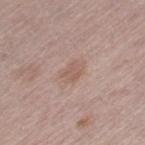Clinical impression: Imaged during a routine full-body skin examination; the lesion was not biopsied and no histopathology is available. Acquisition and patient details: The tile uses white-light illumination. Automated tile analysis of the lesion measured a footprint of about 4.5 mm², a shape eccentricity near 0.7, and a symmetry-axis asymmetry near 0.25. And it measured a detector confidence of about 100 out of 100 that the crop contains a lesion. Located on the left thigh. A male patient, roughly 65 years of age. About 3 mm across. A close-up tile cropped from a whole-body skin photograph, about 15 mm across.